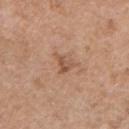No biopsy was performed on this lesion — it was imaged during a full skin examination and was not determined to be concerning.
From the chest.
This is a white-light tile.
Automated tile analysis of the lesion measured a lesion area of about 3 mm², a shape eccentricity near 0.65, and a symmetry-axis asymmetry near 0.45. It also reported a mean CIELAB color near L≈53 a*≈21 b*≈31, roughly 9 lightness units darker than nearby skin, and a normalized lesion–skin contrast near 6.5.
A male patient, in their mid- to late 50s.
Measured at roughly 2.5 mm in maximum diameter.
A region of skin cropped from a whole-body photographic capture, roughly 15 mm wide.